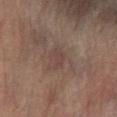The lesion was photographed on a routine skin check and not biopsied; there is no pathology result. A male subject approximately 70 years of age. The tile uses cross-polarized illumination. Located on the right lower leg. A roughly 15 mm field-of-view crop from a total-body skin photograph. Approximately 2.5 mm at its widest. Automated tile analysis of the lesion measured a classifier nevus-likeness of about 0/100.